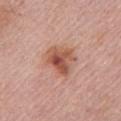Q: Is there a histopathology result?
A: imaged on a skin check; not biopsied
Q: How was the tile lit?
A: white-light
Q: Lesion size?
A: ≈4 mm
Q: Where on the body is the lesion?
A: the left upper arm
Q: Who is the patient?
A: female, aged 63–67
Q: What did automated image analysis measure?
A: a border-irregularity rating of about 3/10, internal color variation of about 8 on a 0–10 scale, and radial color variation of about 2.5; a nevus-likeness score of about 80/100 and a detector confidence of about 100 out of 100 that the crop contains a lesion
Q: How was this image acquired?
A: ~15 mm crop, total-body skin-cancer survey